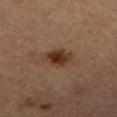biopsy_status: not biopsied; imaged during a skin examination
lighting: cross-polarized
site: leg
patient:
  sex: male
  age_approx: 85+
image:
  source: total-body photography crop
  field_of_view_mm: 15
lesion_size:
  long_diameter_mm_approx: 3.5